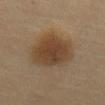* follow-up · catalogued during a skin exam; not biopsied
* TBP lesion metrics · a footprint of about 22 mm² and a shape-asymmetry score of about 0.15 (0 = symmetric); border irregularity of about 1.5 on a 0–10 scale, internal color variation of about 3.5 on a 0–10 scale, and peripheral color asymmetry of about 1; a classifier nevus-likeness of about 100/100
* subject · aged 68 to 72
* acquisition · 15 mm crop, total-body photography
* site · the abdomen